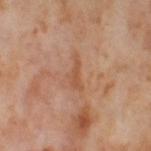{"biopsy_status": "not biopsied; imaged during a skin examination", "patient": {"sex": "female", "age_approx": 55}, "automated_metrics": {"area_mm2_approx": 3.5, "eccentricity": 0.9, "shape_asymmetry": 0.55, "cielab_L": 54, "cielab_a": 23, "cielab_b": 34, "vs_skin_darker_L": 7.0, "vs_skin_contrast_norm": 5.5, "border_irregularity_0_10": 6.5, "color_variation_0_10": 1.0, "peripheral_color_asymmetry": 0.0}, "lesion_size": {"long_diameter_mm_approx": 4.0}, "site": "right thigh", "lighting": "cross-polarized", "image": {"source": "total-body photography crop", "field_of_view_mm": 15}}